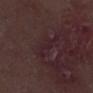{"site": "leg", "patient": {"sex": "male", "age_approx": 65}, "image": {"source": "total-body photography crop", "field_of_view_mm": 15}, "diagnosis": {"histopathology": "actinic keratosis", "malignancy": "indeterminate", "taxonomic_path": ["Indeterminate", "Indeterminate epidermal proliferations", "Solar or actinic keratosis"]}}